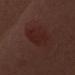Q: Was a biopsy performed?
A: no biopsy performed (imaged during a skin exam)
Q: What are the patient's age and sex?
A: female, roughly 50 years of age
Q: What is the anatomic site?
A: the front of the torso
Q: What is the imaging modality?
A: ~15 mm tile from a whole-body skin photo
Q: Illumination type?
A: white-light illumination
Q: What is the lesion's diameter?
A: about 6 mm
Q: What did automated image analysis measure?
A: a lesion area of about 10 mm², a shape eccentricity near 0.95, and two-axis asymmetry of about 0.3; a lesion color around L≈23 a*≈21 b*≈21 in CIELAB, roughly 5 lightness units darker than nearby skin, and a normalized border contrast of about 6; a nevus-likeness score of about 20/100 and a detector confidence of about 80 out of 100 that the crop contains a lesion